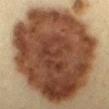* notes: no biopsy performed (imaged during a skin exam)
* subject: male, roughly 35 years of age
* location: the mid back
* image source: total-body-photography crop, ~15 mm field of view